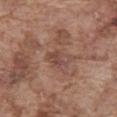Captured during whole-body skin photography for melanoma surveillance; the lesion was not biopsied.
Located on the abdomen.
A male subject, in their mid- to late 70s.
A 15 mm close-up extracted from a 3D total-body photography capture.
Captured under white-light illumination.
Automated image analysis of the tile measured a footprint of about 5 mm² and two-axis asymmetry of about 0.25.
Longest diameter approximately 3 mm.